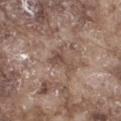Q: Is there a histopathology result?
A: total-body-photography surveillance lesion; no biopsy
Q: Where on the body is the lesion?
A: the right thigh
Q: What kind of image is this?
A: 15 mm crop, total-body photography
Q: Lesion size?
A: ~3 mm (longest diameter)
Q: What are the patient's age and sex?
A: male, aged around 75
Q: Illumination type?
A: white-light